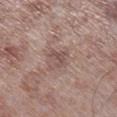Assessment:
Imaged during a routine full-body skin examination; the lesion was not biopsied and no histopathology is available.
Clinical summary:
A region of skin cropped from a whole-body photographic capture, roughly 15 mm wide. The lesion is on the right lower leg. A male patient, aged approximately 55. Imaged with white-light lighting.